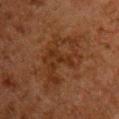Recorded during total-body skin imaging; not selected for excision or biopsy. The subject is a male aged approximately 60. This is a cross-polarized tile. Measured at roughly 8.5 mm in maximum diameter. Cropped from a whole-body photographic skin survey; the tile spans about 15 mm. The lesion is located on the chest. An algorithmic analysis of the crop reported a lesion area of about 24 mm², an outline eccentricity of about 0.85 (0 = round, 1 = elongated), and two-axis asymmetry of about 0.4. The analysis additionally found a classifier nevus-likeness of about 0/100 and a lesion-detection confidence of about 100/100.